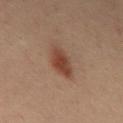Case summary:
• acquisition · ~15 mm crop, total-body skin-cancer survey
• lighting · cross-polarized
• patient · male, aged around 40
• location · the back
• automated lesion analysis · a footprint of about 8 mm², an eccentricity of roughly 0.8, and a symmetry-axis asymmetry near 0.2; a lesion color around L≈34 a*≈17 b*≈23 in CIELAB and about 9 CIELAB-L* units darker than the surrounding skin
• lesion diameter · ~4 mm (longest diameter)